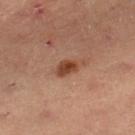Captured during whole-body skin photography for melanoma surveillance; the lesion was not biopsied. From the right lower leg. A 15 mm close-up extracted from a 3D total-body photography capture. A male patient aged 53 to 57.